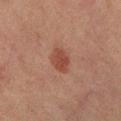This lesion was catalogued during total-body skin photography and was not selected for biopsy. On the right lower leg. A 15 mm crop from a total-body photograph taken for skin-cancer surveillance. The subject is a female approximately 60 years of age.